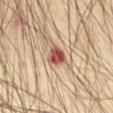notes=catalogued during a skin exam; not biopsied | acquisition=total-body-photography crop, ~15 mm field of view | patient=male, in their mid- to late 70s | tile lighting=cross-polarized | anatomic site=the front of the torso | lesion diameter=~2.5 mm (longest diameter).